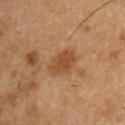The lesion was tiled from a total-body skin photograph and was not biopsied.
This is a cross-polarized tile.
About 4 mm across.
A 15 mm close-up tile from a total-body photography series done for melanoma screening.
The lesion is located on the chest.
A male subject, in their mid- to late 50s.